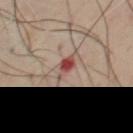This lesion was catalogued during total-body skin photography and was not selected for biopsy. This image is a 15 mm lesion crop taken from a total-body photograph. The patient is a male aged around 55. The lesion is on the chest.